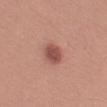Impression: The lesion was photographed on a routine skin check and not biopsied; there is no pathology result. Acquisition and patient details: A lesion tile, about 15 mm wide, cut from a 3D total-body photograph. Longest diameter approximately 3.5 mm. This is a white-light tile. Located on the left upper arm. A male patient approximately 30 years of age.